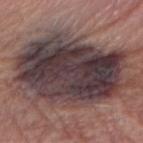workup = imaged on a skin check; not biopsied | image-analysis metrics = a lesion color around L≈38 a*≈15 b*≈14 in CIELAB, about 16 CIELAB-L* units darker than the surrounding skin, and a normalized border contrast of about 14; a border-irregularity index near 4.5/10 and peripheral color asymmetry of about 3; a lesion-detection confidence of about 65/100 | lighting = white-light | imaging modality = total-body-photography crop, ~15 mm field of view | location = the right forearm | diameter = ~14 mm (longest diameter) | subject = male, approximately 70 years of age.